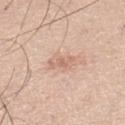Recorded during total-body skin imaging; not selected for excision or biopsy.
The lesion's longest dimension is about 3 mm.
From the left lower leg.
The subject is a male aged approximately 35.
Automated tile analysis of the lesion measured a lesion area of about 4 mm², a shape eccentricity near 0.9, and a symmetry-axis asymmetry near 0.35. The analysis additionally found a border-irregularity rating of about 4.5/10, a within-lesion color-variation index near 1.5/10, and peripheral color asymmetry of about 0.5.
This image is a 15 mm lesion crop taken from a total-body photograph.
The tile uses white-light illumination.